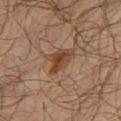Longest diameter approximately 3.5 mm. The patient is a male approximately 65 years of age. Imaged with cross-polarized lighting. A lesion tile, about 15 mm wide, cut from a 3D total-body photograph. From the right thigh.